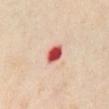{
  "biopsy_status": "not biopsied; imaged during a skin examination",
  "site": "abdomen",
  "automated_metrics": {
    "area_mm2_approx": 4.5,
    "shape_asymmetry": 0.15,
    "cielab_L": 55,
    "cielab_a": 39,
    "cielab_b": 31,
    "vs_skin_darker_L": 22.0,
    "vs_skin_contrast_norm": 13.5
  },
  "lighting": "cross-polarized",
  "image": {
    "source": "total-body photography crop",
    "field_of_view_mm": 15
  },
  "patient": {
    "sex": "male",
    "age_approx": 65
  }
}